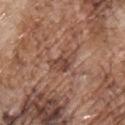* biopsy status · catalogued during a skin exam; not biopsied
* imaging modality · total-body-photography crop, ~15 mm field of view
* lighting · white-light
* anatomic site · the chest
* TBP lesion metrics · a lesion color around L≈45 a*≈20 b*≈27 in CIELAB, about 9 CIELAB-L* units darker than the surrounding skin, and a normalized border contrast of about 7; a border-irregularity rating of about 4/10, internal color variation of about 3.5 on a 0–10 scale, and radial color variation of about 1
* subject · male, aged 68–72
* lesion size · about 4 mm